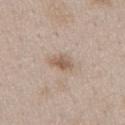This lesion was catalogued during total-body skin photography and was not selected for biopsy. The lesion's longest dimension is about 2.5 mm. A male patient aged approximately 50. The total-body-photography lesion software estimated an average lesion color of about L≈57 a*≈15 b*≈27 (CIELAB). It also reported a classifier nevus-likeness of about 55/100 and a detector confidence of about 100 out of 100 that the crop contains a lesion. This image is a 15 mm lesion crop taken from a total-body photograph. This is a white-light tile. On the chest.